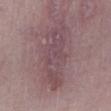Clinical impression:
The lesion was tiled from a total-body skin photograph and was not biopsied.
Image and clinical context:
From the right lower leg. A roughly 15 mm field-of-view crop from a total-body skin photograph. A female subject in their 60s.